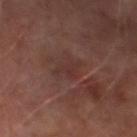Imaged during a routine full-body skin examination; the lesion was not biopsied and no histopathology is available. Longest diameter approximately 3.5 mm. A 15 mm close-up extracted from a 3D total-body photography capture. A male patient about 65 years old. This is a cross-polarized tile. The lesion is located on the left lower leg.